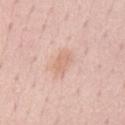  biopsy_status: not biopsied; imaged during a skin examination
  patient:
    sex: male
    age_approx: 60
  site: front of the torso
  lesion_size:
    long_diameter_mm_approx: 3.0
  automated_metrics:
    area_mm2_approx: 3.5
    shape_asymmetry: 0.4
    cielab_L: 68
    cielab_a: 20
    cielab_b: 30
    vs_skin_darker_L: 7.0
    vs_skin_contrast_norm: 5.0
  lighting: white-light
  image:
    source: total-body photography crop
    field_of_view_mm: 15